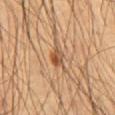Clinical impression:
This lesion was catalogued during total-body skin photography and was not selected for biopsy.
Clinical summary:
From the abdomen. Automated image analysis of the tile measured an area of roughly 3.5 mm², a shape eccentricity near 0.5, and a shape-asymmetry score of about 0.25 (0 = symmetric). The software also gave an average lesion color of about L≈49 a*≈21 b*≈35 (CIELAB), a lesion–skin lightness drop of about 11, and a normalized border contrast of about 8. It also reported internal color variation of about 6 on a 0–10 scale and peripheral color asymmetry of about 2. It also reported a nevus-likeness score of about 90/100 and a lesion-detection confidence of about 100/100. The subject is a male aged approximately 55. This image is a 15 mm lesion crop taken from a total-body photograph.